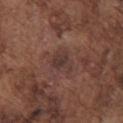Part of a total-body skin-imaging series; this lesion was reviewed on a skin check and was not flagged for biopsy. An algorithmic analysis of the crop reported an average lesion color of about L≈35 a*≈18 b*≈21 (CIELAB), about 7 CIELAB-L* units darker than the surrounding skin, and a normalized lesion–skin contrast near 6.5. The analysis additionally found a border-irregularity index near 4/10, a within-lesion color-variation index near 2/10, and radial color variation of about 0.5. The analysis additionally found a lesion-detection confidence of about 100/100. The patient is a male about 75 years old. A region of skin cropped from a whole-body photographic capture, roughly 15 mm wide. From the chest. Captured under white-light illumination.